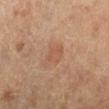Impression:
Captured during whole-body skin photography for melanoma surveillance; the lesion was not biopsied.
Background:
Cropped from a whole-body photographic skin survey; the tile spans about 15 mm. An algorithmic analysis of the crop reported a footprint of about 5 mm², an outline eccentricity of about 0.6 (0 = round, 1 = elongated), and a shape-asymmetry score of about 0.25 (0 = symmetric). It also reported a mean CIELAB color near L≈52 a*≈20 b*≈31, a lesion–skin lightness drop of about 6, and a normalized border contrast of about 4.5. The analysis additionally found a border-irregularity rating of about 2.5/10 and peripheral color asymmetry of about 1. The software also gave a nevus-likeness score of about 15/100 and lesion-presence confidence of about 100/100. A female patient approximately 70 years of age. Approximately 2.5 mm at its widest. The tile uses cross-polarized illumination. From the leg.